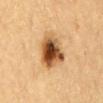follow-up=total-body-photography surveillance lesion; no biopsy
location=the mid back
subject=male, in their mid-80s or older
image=total-body-photography crop, ~15 mm field of view